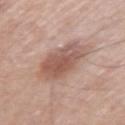notes = catalogued during a skin exam; not biopsied
lesion size = ≈5.5 mm
patient = female, aged 58 to 62
anatomic site = the left upper arm
image source = ~15 mm crop, total-body skin-cancer survey